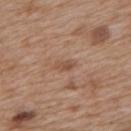follow-up: imaged on a skin check; not biopsied
lighting: white-light
image: total-body-photography crop, ~15 mm field of view
automated lesion analysis: an area of roughly 3.5 mm² and a shape eccentricity near 0.85; a border-irregularity index near 2.5/10 and radial color variation of about 1
subject: female, aged 38–42
anatomic site: the upper back
lesion diameter: ≈2.5 mm